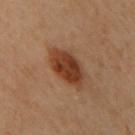Q: Was this lesion biopsied?
A: no biopsy performed (imaged during a skin exam)
Q: How was this image acquired?
A: 15 mm crop, total-body photography
Q: What is the lesion's diameter?
A: about 5 mm
Q: Patient demographics?
A: female, about 60 years old
Q: What is the anatomic site?
A: the upper back
Q: What did automated image analysis measure?
A: an average lesion color of about L≈33 a*≈20 b*≈28 (CIELAB), roughly 11 lightness units darker than nearby skin, and a normalized border contrast of about 10.5; a border-irregularity index near 1.5/10 and a color-variation rating of about 4/10; an automated nevus-likeness rating near 100 out of 100
Q: Illumination type?
A: cross-polarized illumination